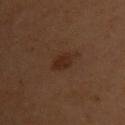biopsy_status: not biopsied; imaged during a skin examination
image:
  source: total-body photography crop
  field_of_view_mm: 15
site: chest
patient:
  sex: female
  age_approx: 50
lesion_size:
  long_diameter_mm_approx: 3.0
automated_metrics:
  vs_skin_darker_L: 6.0
  vs_skin_contrast_norm: 7.0
  border_irregularity_0_10: 1.5
  color_variation_0_10: 1.5
  peripheral_color_asymmetry: 0.5
lighting: cross-polarized